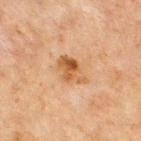Approximately 4 mm at its widest. A male subject, in their mid- to late 60s. This is a cross-polarized tile. A close-up tile cropped from a whole-body skin photograph, about 15 mm across. Located on the front of the torso.